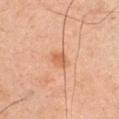A roughly 15 mm field-of-view crop from a total-body skin photograph. A male subject aged around 45. Automated tile analysis of the lesion measured a lesion area of about 3.5 mm², a shape eccentricity near 0.55, and two-axis asymmetry of about 0.3. The software also gave a lesion color around L≈58 a*≈24 b*≈37 in CIELAB, a lesion–skin lightness drop of about 9, and a lesion-to-skin contrast of about 7 (normalized; higher = more distinct). It also reported border irregularity of about 2.5 on a 0–10 scale, internal color variation of about 2.5 on a 0–10 scale, and a peripheral color-asymmetry measure near 1. On the arm. This is a cross-polarized tile. About 2.5 mm across.